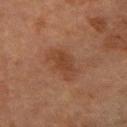The lesion was tiled from a total-body skin photograph and was not biopsied. The lesion is located on the right forearm. A lesion tile, about 15 mm wide, cut from a 3D total-body photograph. The subject is a female aged 53 to 57.